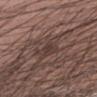size=≈3 mm | patient=male, aged 23–27 | tile lighting=white-light illumination | site=the right upper arm | image=15 mm crop, total-body photography.